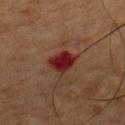Case summary:
– biopsy status: catalogued during a skin exam; not biopsied
– body site: the chest
– patient: male, aged 53 to 57
– lighting: cross-polarized
– image source: total-body-photography crop, ~15 mm field of view